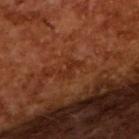notes: total-body-photography surveillance lesion; no biopsy
subject: male, in their mid- to late 60s
diameter: ~3 mm (longest diameter)
lighting: cross-polarized illumination
image-analysis metrics: an area of roughly 3 mm², an outline eccentricity of about 0.85 (0 = round, 1 = elongated), and a shape-asymmetry score of about 0.5 (0 = symmetric)
imaging modality: 15 mm crop, total-body photography